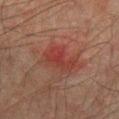Clinical impression: Captured during whole-body skin photography for melanoma surveillance; the lesion was not biopsied. Context: From the abdomen. Captured under cross-polarized illumination. About 4.5 mm across. A male patient about 75 years old. A roughly 15 mm field-of-view crop from a total-body skin photograph. The lesion-visualizer software estimated an area of roughly 9 mm², a shape eccentricity near 0.8, and a shape-asymmetry score of about 0.4 (0 = symmetric). The analysis additionally found about 6 CIELAB-L* units darker than the surrounding skin and a normalized lesion–skin contrast near 6. It also reported internal color variation of about 4.5 on a 0–10 scale and radial color variation of about 1.5. It also reported a classifier nevus-likeness of about 5/100.